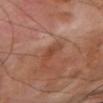workup: catalogued during a skin exam; not biopsied | TBP lesion metrics: an area of roughly 3 mm², an outline eccentricity of about 0.9 (0 = round, 1 = elongated), and a symmetry-axis asymmetry near 0.3; internal color variation of about 0 on a 0–10 scale | lighting: cross-polarized illumination | image source: 15 mm crop, total-body photography | lesion size: ≈3 mm | body site: the right upper arm | patient: male, aged 68 to 72.